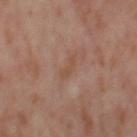Clinical impression: This lesion was catalogued during total-body skin photography and was not selected for biopsy. Clinical summary: A lesion tile, about 15 mm wide, cut from a 3D total-body photograph. Automated tile analysis of the lesion measured a lesion color around L≈50 a*≈19 b*≈29 in CIELAB, a lesion–skin lightness drop of about 5, and a lesion-to-skin contrast of about 5 (normalized; higher = more distinct). The analysis additionally found a nevus-likeness score of about 0/100 and a detector confidence of about 100 out of 100 that the crop contains a lesion. The lesion is on the right thigh. Approximately 2.5 mm at its widest. A female patient, approximately 55 years of age. Imaged with cross-polarized lighting.